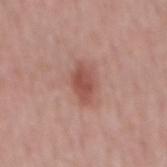{"biopsy_status": "not biopsied; imaged during a skin examination", "automated_metrics": {"cielab_L": 51, "cielab_a": 25, "cielab_b": 26, "vs_skin_darker_L": 10.0, "lesion_detection_confidence_0_100": 100}, "site": "mid back", "patient": {"sex": "male", "age_approx": 50}, "image": {"source": "total-body photography crop", "field_of_view_mm": 15}, "lighting": "white-light"}